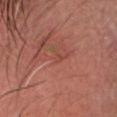<tbp_lesion>
<biopsy_status>not biopsied; imaged during a skin examination</biopsy_status>
<lesion_size>
  <long_diameter_mm_approx>1.0</long_diameter_mm_approx>
</lesion_size>
<automated_metrics>
  <area_mm2_approx>1.0</area_mm2_approx>
  <eccentricity>0.65</eccentricity>
  <shape_asymmetry>0.35</shape_asymmetry>
  <border_irregularity_0_10>2.5</border_irregularity_0_10>
  <color_variation_0_10>0.0</color_variation_0_10>
</automated_metrics>
<image>
  <source>total-body photography crop</source>
  <field_of_view_mm>15</field_of_view_mm>
</image>
<site>head or neck</site>
<patient>
  <sex>male</sex>
  <age_approx>50</age_approx>
</patient>
</tbp_lesion>